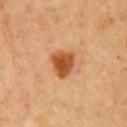- follow-up · no biopsy performed (imaged during a skin exam)
- body site · the chest
- size · ~3.5 mm (longest diameter)
- image-analysis metrics · an outline eccentricity of about 0.5 (0 = round, 1 = elongated) and two-axis asymmetry of about 0.3; a border-irregularity index near 2.5/10 and radial color variation of about 1; a nevus-likeness score of about 100/100 and a detector confidence of about 100 out of 100 that the crop contains a lesion
- patient · male, aged approximately 50
- imaging modality · ~15 mm tile from a whole-body skin photo
- illumination · cross-polarized